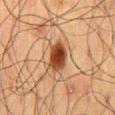biopsy_status: not biopsied; imaged during a skin examination
patient:
  sex: male
  age_approx: 60
lesion_size:
  long_diameter_mm_approx: 4.0
automated_metrics:
  area_mm2_approx: 8.5
  eccentricity: 0.8
  shape_asymmetry: 0.2
  vs_skin_darker_L: 15.0
site: mid back
lighting: cross-polarized
image:
  source: total-body photography crop
  field_of_view_mm: 15